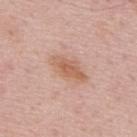Impression: Part of a total-body skin-imaging series; this lesion was reviewed on a skin check and was not flagged for biopsy. Background: This image is a 15 mm lesion crop taken from a total-body photograph. The lesion is located on the upper back. A male patient in their 50s. Captured under white-light illumination.